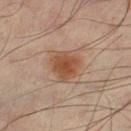workup = no biopsy performed (imaged during a skin exam)
illumination = cross-polarized
lesion size = about 3.5 mm
subject = male, aged around 55
image-analysis metrics = border irregularity of about 2 on a 0–10 scale, a within-lesion color-variation index near 3.5/10, and a peripheral color-asymmetry measure near 1
image = 15 mm crop, total-body photography
body site = the left lower leg